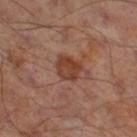Part of a total-body skin-imaging series; this lesion was reviewed on a skin check and was not flagged for biopsy.
A region of skin cropped from a whole-body photographic capture, roughly 15 mm wide.
The lesion is on the right thigh.
Automated image analysis of the tile measured a lesion color around L≈38 a*≈22 b*≈28 in CIELAB, roughly 9 lightness units darker than nearby skin, and a normalized lesion–skin contrast near 8.5.
Captured under cross-polarized illumination.
The lesion's longest dimension is about 3 mm.
A male subject approximately 65 years of age.